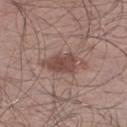Recorded during total-body skin imaging; not selected for excision or biopsy.